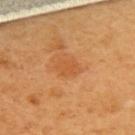biopsy status = imaged on a skin check; not biopsied | lesion size = ≈3 mm | acquisition = 15 mm crop, total-body photography | automated metrics = a border-irregularity rating of about 2/10, a color-variation rating of about 1.5/10, and radial color variation of about 0.5 | anatomic site = the upper back | illumination = cross-polarized illumination | patient = female, aged around 55.